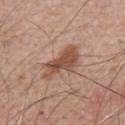biopsy_status: not biopsied; imaged during a skin examination
lesion_size:
  long_diameter_mm_approx: 5.5
lighting: white-light
image:
  source: total-body photography crop
  field_of_view_mm: 15
site: front of the torso
patient:
  sex: male
  age_approx: 65